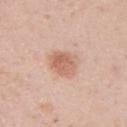Context: Longest diameter approximately 3.5 mm. Captured under white-light illumination. A female subject, aged 28 to 32. Located on the right upper arm. A close-up tile cropped from a whole-body skin photograph, about 15 mm across.